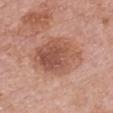{
  "automated_metrics": {
    "area_mm2_approx": 23.0,
    "eccentricity": 0.65,
    "shape_asymmetry": 0.2,
    "border_irregularity_0_10": 2.5,
    "color_variation_0_10": 5.5,
    "peripheral_color_asymmetry": 2.0,
    "nevus_likeness_0_100": 20,
    "lesion_detection_confidence_0_100": 100
  },
  "lighting": "white-light",
  "patient": {
    "sex": "female",
    "age_approx": 60
  },
  "lesion_size": {
    "long_diameter_mm_approx": 6.0
  },
  "site": "front of the torso",
  "image": {
    "source": "total-body photography crop",
    "field_of_view_mm": 15
  }
}